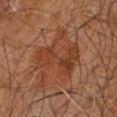Acquisition and patient details:
Longest diameter approximately 6 mm. The tile uses cross-polarized illumination. Cropped from a whole-body photographic skin survey; the tile spans about 15 mm. Located on the right leg. A male subject, about 60 years old. Automated image analysis of the tile measured a lesion area of about 15 mm², an outline eccentricity of about 0.85 (0 = round, 1 = elongated), and a shape-asymmetry score of about 0.4 (0 = symmetric). The analysis additionally found a border-irregularity index near 6/10, a within-lesion color-variation index near 4.5/10, and radial color variation of about 2. The analysis additionally found a classifier nevus-likeness of about 0/100 and lesion-presence confidence of about 100/100.